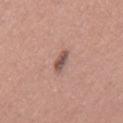Clinical impression:
No biopsy was performed on this lesion — it was imaged during a full skin examination and was not determined to be concerning.
Clinical summary:
A 15 mm crop from a total-body photograph taken for skin-cancer surveillance. On the back. About 3 mm across. A male patient, aged approximately 35.